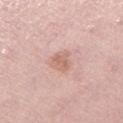No biopsy was performed on this lesion — it was imaged during a full skin examination and was not determined to be concerning. The lesion's longest dimension is about 3 mm. The lesion-visualizer software estimated an area of roughly 5 mm² and an eccentricity of roughly 0.5. The analysis additionally found a border-irregularity rating of about 2.5/10, a color-variation rating of about 2/10, and peripheral color asymmetry of about 0.5. The software also gave an automated nevus-likeness rating near 5 out of 100 and a lesion-detection confidence of about 100/100. Located on the left thigh. A female patient in their mid-60s. Cropped from a total-body skin-imaging series; the visible field is about 15 mm.